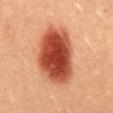biopsy status=total-body-photography surveillance lesion; no biopsy | site=the abdomen | patient=male, aged approximately 40 | image=15 mm crop, total-body photography | image-analysis metrics=an average lesion color of about L≈40 a*≈28 b*≈30 (CIELAB) and a normalized border contrast of about 12.5; a peripheral color-asymmetry measure near 1.5; a detector confidence of about 100 out of 100 that the crop contains a lesion.